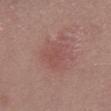A male subject, roughly 40 years of age. Longest diameter approximately 4.5 mm. A 15 mm crop from a total-body photograph taken for skin-cancer surveillance. On the back.